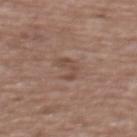Q: Was this lesion biopsied?
A: catalogued during a skin exam; not biopsied
Q: Who is the patient?
A: female, in their mid-70s
Q: What did automated image analysis measure?
A: a footprint of about 4 mm² and an eccentricity of roughly 0.9; a lesion color around L≈47 a*≈18 b*≈25 in CIELAB and a normalized border contrast of about 5.5; an automated nevus-likeness rating near 0 out of 100
Q: What is the imaging modality?
A: 15 mm crop, total-body photography
Q: How large is the lesion?
A: about 3.5 mm
Q: Illumination type?
A: white-light
Q: What is the anatomic site?
A: the upper back